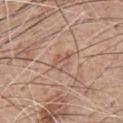Recorded during total-body skin imaging; not selected for excision or biopsy. A roughly 15 mm field-of-view crop from a total-body skin photograph. The subject is a male in their 60s. The lesion is located on the chest.